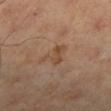biopsy status=total-body-photography surveillance lesion; no biopsy
location=the leg
size=≈3 mm
image=15 mm crop, total-body photography
patient=male, in their mid-40s
tile lighting=cross-polarized illumination
image-analysis metrics=an area of roughly 5 mm²